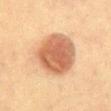Part of a total-body skin-imaging series; this lesion was reviewed on a skin check and was not flagged for biopsy. The tile uses cross-polarized illumination. The recorded lesion diameter is about 6 mm. A 15 mm close-up tile from a total-body photography series done for melanoma screening. The lesion is on the abdomen. A female subject about 50 years old.